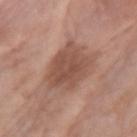<case>
  <biopsy_status>not biopsied; imaged during a skin examination</biopsy_status>
  <image>
    <source>total-body photography crop</source>
    <field_of_view_mm>15</field_of_view_mm>
  </image>
  <site>right upper arm</site>
  <lesion_size>
    <long_diameter_mm_approx>5.5</long_diameter_mm_approx>
  </lesion_size>
  <patient>
    <sex>female</sex>
    <age_approx>85+</age_approx>
  </patient>
  <automated_metrics>
    <area_mm2_approx>19.0</area_mm2_approx>
    <eccentricity>0.55</eccentricity>
    <shape_asymmetry>0.2</shape_asymmetry>
    <nevus_likeness_0_100>10</nevus_likeness_0_100>
    <lesion_detection_confidence_0_100>100</lesion_detection_confidence_0_100>
  </automated_metrics>
  <lighting>white-light</lighting>
</case>